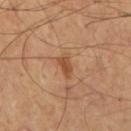Clinical impression: Imaged during a routine full-body skin examination; the lesion was not biopsied and no histopathology is available. Background: Measured at roughly 3 mm in maximum diameter. The tile uses cross-polarized illumination. This image is a 15 mm lesion crop taken from a total-body photograph. A male patient in their mid- to late 50s. The total-body-photography lesion software estimated a border-irregularity rating of about 3.5/10 and radial color variation of about 1. The software also gave a classifier nevus-likeness of about 55/100 and a detector confidence of about 100 out of 100 that the crop contains a lesion. The lesion is located on the mid back.